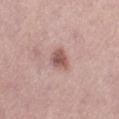notes: total-body-photography surveillance lesion; no biopsy
patient: female, aged around 60
automated lesion analysis: an area of roughly 5 mm², a shape eccentricity near 0.6, and a symmetry-axis asymmetry near 0.25; a lesion-to-skin contrast of about 8 (normalized; higher = more distinct); a border-irregularity index near 2/10, a color-variation rating of about 3/10, and radial color variation of about 1
location: the left thigh
imaging modality: total-body-photography crop, ~15 mm field of view
lesion size: ≈3 mm
illumination: white-light illumination